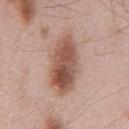Q: Is there a histopathology result?
A: total-body-photography surveillance lesion; no biopsy
Q: What kind of image is this?
A: 15 mm crop, total-body photography
Q: Where on the body is the lesion?
A: the chest
Q: How was the tile lit?
A: white-light illumination
Q: Automated lesion metrics?
A: an area of roughly 22 mm², a shape eccentricity near 0.9, and two-axis asymmetry of about 0.15; an average lesion color of about L≈54 a*≈21 b*≈27 (CIELAB), roughly 14 lightness units darker than nearby skin, and a normalized lesion–skin contrast near 9; internal color variation of about 7.5 on a 0–10 scale; a nevus-likeness score of about 90/100
Q: What are the patient's age and sex?
A: male, roughly 55 years of age